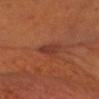biopsy status: catalogued during a skin exam; not biopsied
patient: male, aged around 60
automated lesion analysis: a mean CIELAB color near L≈35 a*≈25 b*≈28, a lesion–skin lightness drop of about 7, and a normalized border contrast of about 7
anatomic site: the head or neck
acquisition: 15 mm crop, total-body photography